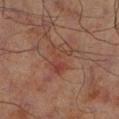The patient is a male approximately 70 years of age.
On the left lower leg.
Automated tile analysis of the lesion measured a nevus-likeness score of about 0/100 and lesion-presence confidence of about 100/100.
Longest diameter approximately 3.5 mm.
Imaged with cross-polarized lighting.
Cropped from a total-body skin-imaging series; the visible field is about 15 mm.